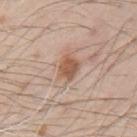{"biopsy_status": "not biopsied; imaged during a skin examination", "lighting": "white-light", "site": "left upper arm", "patient": {"sex": "male", "age_approx": 70}, "lesion_size": {"long_diameter_mm_approx": 3.0}, "automated_metrics": {"cielab_L": 56, "cielab_a": 19, "cielab_b": 30, "vs_skin_contrast_norm": 8.0, "color_variation_0_10": 2.5, "peripheral_color_asymmetry": 1.0}, "image": {"source": "total-body photography crop", "field_of_view_mm": 15}}